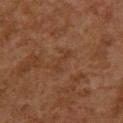Assessment:
Recorded during total-body skin imaging; not selected for excision or biopsy.
Acquisition and patient details:
A 15 mm crop from a total-body photograph taken for skin-cancer surveillance. A female subject, about 60 years old. Measured at roughly 3 mm in maximum diameter. On the upper back.